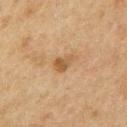Notes:
– notes · total-body-photography surveillance lesion; no biopsy
– size · about 2.5 mm
– patient · male, aged approximately 75
– image source · 15 mm crop, total-body photography
– anatomic site · the front of the torso